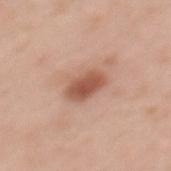– workup · catalogued during a skin exam; not biopsied
– subject · female, aged approximately 50
– anatomic site · the mid back
– tile lighting · white-light illumination
– size · ~4 mm (longest diameter)
– imaging modality · total-body-photography crop, ~15 mm field of view